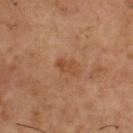This lesion was catalogued during total-body skin photography and was not selected for biopsy.
A male patient, aged 53 to 57.
An algorithmic analysis of the crop reported a footprint of about 3.5 mm². The analysis additionally found an automated nevus-likeness rating near 5 out of 100 and lesion-presence confidence of about 100/100.
Captured under cross-polarized illumination.
Longest diameter approximately 2.5 mm.
Cropped from a whole-body photographic skin survey; the tile spans about 15 mm.
The lesion is located on the upper back.